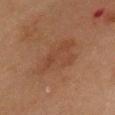{"biopsy_status": "not biopsied; imaged during a skin examination", "image": {"source": "total-body photography crop", "field_of_view_mm": 15}, "lighting": "cross-polarized", "site": "front of the torso", "patient": {"sex": "male", "age_approx": 70}, "lesion_size": {"long_diameter_mm_approx": 6.0}}